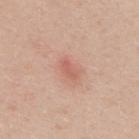Acquisition and patient details: A female patient, roughly 30 years of age. Automated tile analysis of the lesion measured a footprint of about 3.5 mm², an eccentricity of roughly 0.85, and a shape-asymmetry score of about 0.3 (0 = symmetric). Located on the upper back. Cropped from a total-body skin-imaging series; the visible field is about 15 mm. Measured at roughly 2.5 mm in maximum diameter. Imaged with white-light lighting.